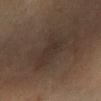• workup — imaged on a skin check; not biopsied
• location — the leg
• image — ~15 mm crop, total-body skin-cancer survey
• size — ≈4.5 mm
• automated lesion analysis — an area of roughly 11 mm², a shape eccentricity near 0.75, and two-axis asymmetry of about 0.35; a border-irregularity index near 4.5/10, internal color variation of about 2.5 on a 0–10 scale, and peripheral color asymmetry of about 1; a classifier nevus-likeness of about 0/100 and a lesion-detection confidence of about 95/100
• subject — female, about 70 years old